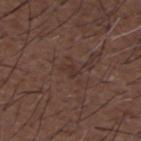notes=total-body-photography surveillance lesion; no biopsy